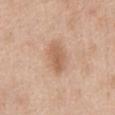{
  "biopsy_status": "not biopsied; imaged during a skin examination",
  "lighting": "white-light",
  "image": {
    "source": "total-body photography crop",
    "field_of_view_mm": 15
  },
  "lesion_size": {
    "long_diameter_mm_approx": 4.0
  },
  "automated_metrics": {
    "vs_skin_darker_L": 10.0,
    "vs_skin_contrast_norm": 6.5,
    "nevus_likeness_0_100": 65,
    "lesion_detection_confidence_0_100": 100
  },
  "patient": {
    "sex": "female",
    "age_approx": 40
  },
  "site": "abdomen"
}